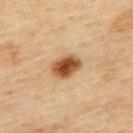image: 15 mm crop, total-body photography | patient: male, about 45 years old | tile lighting: cross-polarized | body site: the upper back | TBP lesion metrics: an area of roughly 8.5 mm², an outline eccentricity of about 0.7 (0 = round, 1 = elongated), and a symmetry-axis asymmetry near 0.1; a lesion–skin lightness drop of about 15 and a lesion-to-skin contrast of about 11.5 (normalized; higher = more distinct); border irregularity of about 1 on a 0–10 scale and peripheral color asymmetry of about 2 | diameter: about 3.5 mm.